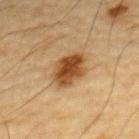{"biopsy_status": "not biopsied; imaged during a skin examination", "image": {"source": "total-body photography crop", "field_of_view_mm": 15}, "site": "chest", "lesion_size": {"long_diameter_mm_approx": 4.0}, "patient": {"sex": "male", "age_approx": "85+"}, "lighting": "cross-polarized"}